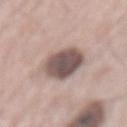Q: Is there a histopathology result?
A: total-body-photography surveillance lesion; no biopsy
Q: What did automated image analysis measure?
A: a lesion color around L≈52 a*≈15 b*≈19 in CIELAB, roughly 17 lightness units darker than nearby skin, and a lesion-to-skin contrast of about 11.5 (normalized; higher = more distinct); border irregularity of about 1.5 on a 0–10 scale, a color-variation rating of about 5/10, and peripheral color asymmetry of about 1.5; an automated nevus-likeness rating near 10 out of 100 and a lesion-detection confidence of about 100/100
Q: Illumination type?
A: white-light
Q: Patient demographics?
A: male, aged around 65
Q: What is the imaging modality?
A: 15 mm crop, total-body photography
Q: What is the lesion's diameter?
A: ~5.5 mm (longest diameter)
Q: Where on the body is the lesion?
A: the mid back